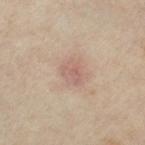Part of a total-body skin-imaging series; this lesion was reviewed on a skin check and was not flagged for biopsy.
Captured under cross-polarized illumination.
The lesion is on the leg.
The subject is a female in their mid- to late 30s.
The lesion's longest dimension is about 3 mm.
The lesion-visualizer software estimated border irregularity of about 2 on a 0–10 scale and peripheral color asymmetry of about 1. And it measured a nevus-likeness score of about 15/100.
A 15 mm crop from a total-body photograph taken for skin-cancer surveillance.